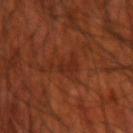  biopsy_status: not biopsied; imaged during a skin examination
  site: right upper arm
  image:
    source: total-body photography crop
    field_of_view_mm: 15
  patient:
    sex: male
    age_approx: 70
  lighting: cross-polarized